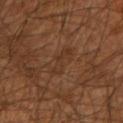The lesion was photographed on a routine skin check and not biopsied; there is no pathology result. About 3.5 mm across. Automated tile analysis of the lesion measured an area of roughly 4 mm², an eccentricity of roughly 0.9, and a shape-asymmetry score of about 0.45 (0 = symmetric). The software also gave internal color variation of about 2 on a 0–10 scale and a peripheral color-asymmetry measure near 0.5. It also reported a classifier nevus-likeness of about 0/100 and a lesion-detection confidence of about 100/100. The lesion is on the arm. A male subject, approximately 45 years of age. A 15 mm close-up tile from a total-body photography series done for melanoma screening.